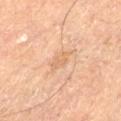Assessment: Imaged during a routine full-body skin examination; the lesion was not biopsied and no histopathology is available. Image and clinical context: Approximately 2.5 mm at its widest. Cropped from a whole-body photographic skin survey; the tile spans about 15 mm. Imaged with cross-polarized lighting. The subject is a male in their mid-60s. From the left thigh.